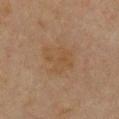Notes:
– follow-up · catalogued during a skin exam; not biopsied
– patient · female, aged around 70
– image source · total-body-photography crop, ~15 mm field of view
– body site · the chest
– lesion diameter · ~4 mm (longest diameter)